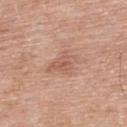Captured during whole-body skin photography for melanoma surveillance; the lesion was not biopsied.
The lesion is on the upper back.
A lesion tile, about 15 mm wide, cut from a 3D total-body photograph.
A male patient, aged 68–72.